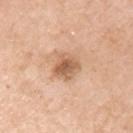The lesion was photographed on a routine skin check and not biopsied; there is no pathology result. A roughly 15 mm field-of-view crop from a total-body skin photograph. The lesion's longest dimension is about 3.5 mm. The tile uses white-light illumination. The patient is a female in their mid- to late 50s. The lesion is on the left upper arm.